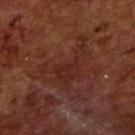Recorded during total-body skin imaging; not selected for excision or biopsy. A roughly 15 mm field-of-view crop from a total-body skin photograph. Automated image analysis of the tile measured a lesion color around L≈27 a*≈23 b*≈24 in CIELAB, about 5 CIELAB-L* units darker than the surrounding skin, and a normalized border contrast of about 5. The software also gave a color-variation rating of about 3/10 and a peripheral color-asymmetry measure near 1. The analysis additionally found lesion-presence confidence of about 80/100. A male subject, aged approximately 65. This is a cross-polarized tile. Approximately 7 mm at its widest.